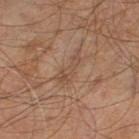Imaged during a routine full-body skin examination; the lesion was not biopsied and no histopathology is available.
The lesion is located on the right leg.
The lesion-visualizer software estimated a footprint of about 5 mm². The analysis additionally found a border-irregularity index near 6.5/10 and a color-variation rating of about 1.5/10.
A male subject roughly 60 years of age.
The lesion's longest dimension is about 4 mm.
Imaged with cross-polarized lighting.
A 15 mm close-up tile from a total-body photography series done for melanoma screening.